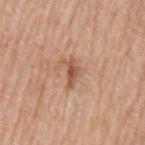Imaged during a routine full-body skin examination; the lesion was not biopsied and no histopathology is available. Imaged with white-light lighting. A male patient, about 70 years old. From the left upper arm. A lesion tile, about 15 mm wide, cut from a 3D total-body photograph. Approximately 3.5 mm at its widest.